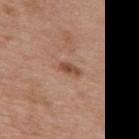Imaged during a routine full-body skin examination; the lesion was not biopsied and no histopathology is available. Measured at roughly 2.5 mm in maximum diameter. A region of skin cropped from a whole-body photographic capture, roughly 15 mm wide. Captured under white-light illumination. From the back. The subject is a female aged approximately 40.